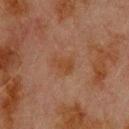biopsy status: no biopsy performed (imaged during a skin exam) | site: the back | patient: male, aged approximately 80 | tile lighting: cross-polarized | image: ~15 mm crop, total-body skin-cancer survey | automated metrics: a footprint of about 5 mm², an eccentricity of roughly 0.75, and a shape-asymmetry score of about 0.3 (0 = symmetric); about 4 CIELAB-L* units darker than the surrounding skin and a lesion-to-skin contrast of about 6.5 (normalized; higher = more distinct); a border-irregularity rating of about 3/10, a within-lesion color-variation index near 1.5/10, and radial color variation of about 0.5; a classifier nevus-likeness of about 5/100 and a lesion-detection confidence of about 100/100 | lesion diameter: about 3 mm.